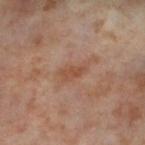Q: Was this lesion biopsied?
A: total-body-photography surveillance lesion; no biopsy
Q: What kind of image is this?
A: ~15 mm crop, total-body skin-cancer survey
Q: What are the patient's age and sex?
A: female, aged 53–57
Q: Where on the body is the lesion?
A: the left thigh
Q: Illumination type?
A: cross-polarized illumination